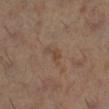Recorded during total-body skin imaging; not selected for excision or biopsy. A female subject, aged around 60. Imaged with cross-polarized lighting. The recorded lesion diameter is about 3 mm. A 15 mm crop from a total-body photograph taken for skin-cancer surveillance. On the right lower leg.